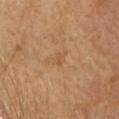follow-up: no biopsy performed (imaged during a skin exam) | lesion diameter: ≈2 mm | tile lighting: cross-polarized | body site: the head or neck | imaging modality: total-body-photography crop, ~15 mm field of view | automated lesion analysis: a mean CIELAB color near L≈54 a*≈20 b*≈37 and a lesion-to-skin contrast of about 4 (normalized; higher = more distinct) | subject: female, approximately 55 years of age.